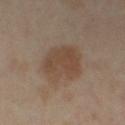Assessment: This lesion was catalogued during total-body skin photography and was not selected for biopsy. Clinical summary: The lesion is on the leg. A female subject, in their 50s. A 15 mm close-up extracted from a 3D total-body photography capture.